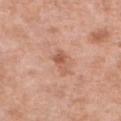This lesion was catalogued during total-body skin photography and was not selected for biopsy. The lesion is located on the front of the torso. The total-body-photography lesion software estimated a border-irregularity index near 4/10, a within-lesion color-variation index near 2.5/10, and radial color variation of about 1. A 15 mm close-up extracted from a 3D total-body photography capture. A female subject, aged 48 to 52. Captured under white-light illumination.